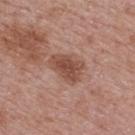The subject is a female aged 63 to 67. About 4 mm across. A 15 mm close-up tile from a total-body photography series done for melanoma screening. On the upper back.